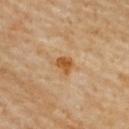Clinical impression:
Part of a total-body skin-imaging series; this lesion was reviewed on a skin check and was not flagged for biopsy.
Context:
The tile uses cross-polarized illumination. The total-body-photography lesion software estimated an area of roughly 3.5 mm² and a shape-asymmetry score of about 0.2 (0 = symmetric). The software also gave a lesion color around L≈55 a*≈24 b*≈43 in CIELAB and a normalized border contrast of about 9.5. The patient is a female aged 68 to 72. A close-up tile cropped from a whole-body skin photograph, about 15 mm across. On the back. The lesion's longest dimension is about 2 mm.